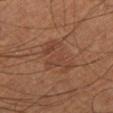Q: Is there a histopathology result?
A: imaged on a skin check; not biopsied
Q: How was this image acquired?
A: ~15 mm crop, total-body skin-cancer survey
Q: What is the lesion's diameter?
A: ~5 mm (longest diameter)
Q: Who is the patient?
A: male, roughly 60 years of age
Q: Illumination type?
A: cross-polarized
Q: Where on the body is the lesion?
A: the left lower leg
Q: What did automated image analysis measure?
A: an outline eccentricity of about 0.8 (0 = round, 1 = elongated) and two-axis asymmetry of about 0.4; lesion-presence confidence of about 100/100